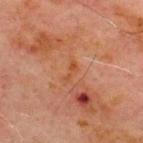Assessment: This lesion was catalogued during total-body skin photography and was not selected for biopsy. Context: Located on the chest. This is a cross-polarized tile. The lesion's longest dimension is about 2.5 mm. A male patient, roughly 70 years of age. A roughly 15 mm field-of-view crop from a total-body skin photograph.